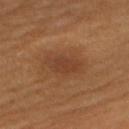The lesion was tiled from a total-body skin photograph and was not biopsied.
Imaged with cross-polarized lighting.
The lesion is on the upper back.
About 4 mm across.
The patient is a female aged 63–67.
A roughly 15 mm field-of-view crop from a total-body skin photograph.